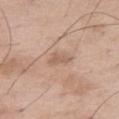Q: Was a biopsy performed?
A: total-body-photography surveillance lesion; no biopsy
Q: How large is the lesion?
A: about 2.5 mm
Q: What are the patient's age and sex?
A: male, approximately 50 years of age
Q: What kind of image is this?
A: 15 mm crop, total-body photography
Q: Where on the body is the lesion?
A: the right thigh
Q: Automated lesion metrics?
A: a lesion area of about 3 mm², an outline eccentricity of about 0.85 (0 = round, 1 = elongated), and a symmetry-axis asymmetry near 0.45; a lesion color around L≈59 a*≈18 b*≈29 in CIELAB, a lesion–skin lightness drop of about 8, and a lesion-to-skin contrast of about 5.5 (normalized; higher = more distinct); a border-irregularity rating of about 4.5/10, a within-lesion color-variation index near 0.5/10, and radial color variation of about 0
Q: Illumination type?
A: white-light illumination